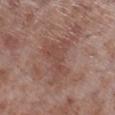<case>
  <biopsy_status>not biopsied; imaged during a skin examination</biopsy_status>
  <site>right lower leg</site>
  <automated_metrics>
    <eccentricity>0.8</eccentricity>
    <shape_asymmetry>0.55</shape_asymmetry>
    <cielab_L>47</cielab_L>
    <cielab_a>21</cielab_a>
    <cielab_b>24</cielab_b>
    <vs_skin_darker_L>7.0</vs_skin_darker_L>
    <vs_skin_contrast_norm>5.5</vs_skin_contrast_norm>
    <border_irregularity_0_10>7.5</border_irregularity_0_10>
    <peripheral_color_asymmetry>0.5</peripheral_color_asymmetry>
  </automated_metrics>
  <lighting>white-light</lighting>
  <patient>
    <sex>male</sex>
    <age_approx>55</age_approx>
  </patient>
  <image>
    <source>total-body photography crop</source>
    <field_of_view_mm>15</field_of_view_mm>
  </image>
  <lesion_size>
    <long_diameter_mm_approx>6.5</long_diameter_mm_approx>
  </lesion_size>
</case>